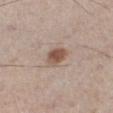This lesion was catalogued during total-body skin photography and was not selected for biopsy.
The tile uses white-light illumination.
From the left lower leg.
An algorithmic analysis of the crop reported a lesion color around L≈52 a*≈17 b*≈27 in CIELAB and a lesion–skin lightness drop of about 12.
A male subject, aged 58–62.
Approximately 3 mm at its widest.
A region of skin cropped from a whole-body photographic capture, roughly 15 mm wide.